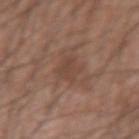Captured during whole-body skin photography for melanoma surveillance; the lesion was not biopsied.
A male patient, aged 28–32.
A close-up tile cropped from a whole-body skin photograph, about 15 mm across.
Located on the left forearm.